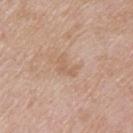biopsy status — catalogued during a skin exam; not biopsied
imaging modality — total-body-photography crop, ~15 mm field of view
lighting — white-light illumination
subject — male, in their 60s
location — the upper back